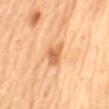Assessment: This lesion was catalogued during total-body skin photography and was not selected for biopsy. Acquisition and patient details: The total-body-photography lesion software estimated border irregularity of about 3 on a 0–10 scale, internal color variation of about 3.5 on a 0–10 scale, and peripheral color asymmetry of about 1. It also reported a nevus-likeness score of about 40/100 and lesion-presence confidence of about 100/100. The patient is a female in their mid- to late 60s. The lesion is located on the mid back. A 15 mm close-up extracted from a 3D total-body photography capture.